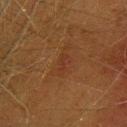Q: Was a biopsy performed?
A: total-body-photography surveillance lesion; no biopsy
Q: What is the imaging modality?
A: 15 mm crop, total-body photography
Q: What is the anatomic site?
A: the upper back
Q: Who is the patient?
A: female, approximately 40 years of age
Q: Illumination type?
A: cross-polarized illumination